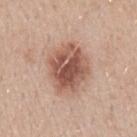{"biopsy_status": "not biopsied; imaged during a skin examination", "image": {"source": "total-body photography crop", "field_of_view_mm": 15}, "patient": {"sex": "male", "age_approx": 30}, "site": "left forearm"}